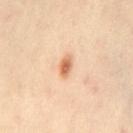acquisition=15 mm crop, total-body photography | anatomic site=the right leg | illumination=cross-polarized illumination | subject=female, roughly 40 years of age | lesion size=≈3 mm.